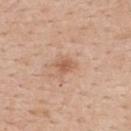Part of a total-body skin-imaging series; this lesion was reviewed on a skin check and was not flagged for biopsy. A 15 mm crop from a total-body photograph taken for skin-cancer surveillance. On the upper back. A female patient approximately 45 years of age. Automated tile analysis of the lesion measured an area of roughly 3.5 mm², an eccentricity of roughly 0.7, and a symmetry-axis asymmetry near 0.25. The analysis additionally found about 9 CIELAB-L* units darker than the surrounding skin and a normalized lesion–skin contrast near 6.5.